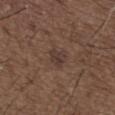<record>
  <biopsy_status>not biopsied; imaged during a skin examination</biopsy_status>
  <patient>
    <sex>male</sex>
    <age_approx>50</age_approx>
  </patient>
  <image>
    <source>total-body photography crop</source>
    <field_of_view_mm>15</field_of_view_mm>
  </image>
  <site>upper back</site>
</record>